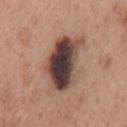Q: Patient demographics?
A: male, aged 53–57
Q: Lesion size?
A: about 7 mm
Q: What kind of image is this?
A: total-body-photography crop, ~15 mm field of view
Q: What lighting was used for the tile?
A: white-light
Q: What is the anatomic site?
A: the chest
Q: Automated lesion metrics?
A: a shape eccentricity near 0.85 and two-axis asymmetry of about 0.25; a mean CIELAB color near L≈41 a*≈17 b*≈21 and a lesion–skin lightness drop of about 20